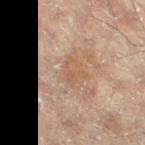Measured at roughly 3 mm in maximum diameter.
A female subject, aged 78 to 82.
The lesion-visualizer software estimated a footprint of about 3.5 mm² and an eccentricity of roughly 0.7.
This is a cross-polarized tile.
A close-up tile cropped from a whole-body skin photograph, about 15 mm across.
The lesion is on the left leg.